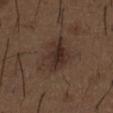This lesion was catalogued during total-body skin photography and was not selected for biopsy. The lesion is on the abdomen. Cropped from a total-body skin-imaging series; the visible field is about 15 mm. This is a white-light tile. A male subject, aged around 50.